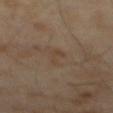notes=catalogued during a skin exam; not biopsied
subject=male, roughly 65 years of age
acquisition=~15 mm tile from a whole-body skin photo
lighting=cross-polarized